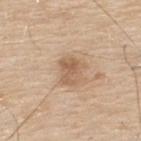- workup — no biopsy performed (imaged during a skin exam)